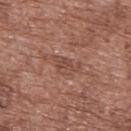Impression: This lesion was catalogued during total-body skin photography and was not selected for biopsy. Context: The total-body-photography lesion software estimated an area of roughly 4.5 mm² and an outline eccentricity of about 0.8 (0 = round, 1 = elongated). The software also gave a border-irregularity index near 6.5/10 and peripheral color asymmetry of about 0.5. About 3.5 mm across. The lesion is located on the upper back. This is a white-light tile. A male subject, aged 73–77. A 15 mm crop from a total-body photograph taken for skin-cancer surveillance.